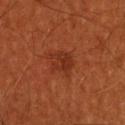Imaged during a routine full-body skin examination; the lesion was not biopsied and no histopathology is available. Cropped from a whole-body photographic skin survey; the tile spans about 15 mm. Located on the head or neck. The total-body-photography lesion software estimated an outline eccentricity of about 0.5 (0 = round, 1 = elongated) and a shape-asymmetry score of about 0.35 (0 = symmetric). And it measured a nevus-likeness score of about 10/100 and a lesion-detection confidence of about 100/100. A male patient roughly 60 years of age.